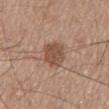Impression: Imaged during a routine full-body skin examination; the lesion was not biopsied and no histopathology is available. Clinical summary: Cropped from a total-body skin-imaging series; the visible field is about 15 mm. From the mid back. Imaged with white-light lighting. The patient is a male in their mid- to late 60s. About 4 mm across. The lesion-visualizer software estimated an area of roughly 8.5 mm², an eccentricity of roughly 0.75, and a symmetry-axis asymmetry near 0.2. It also reported a nevus-likeness score of about 65/100.